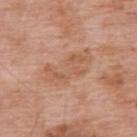Clinical impression: The lesion was tiled from a total-body skin photograph and was not biopsied. Clinical summary: Longest diameter approximately 6.5 mm. The lesion is on the upper back. A 15 mm close-up extracted from a 3D total-body photography capture. The patient is a male aged 58–62.